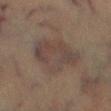Captured during whole-body skin photography for melanoma surveillance; the lesion was not biopsied.
A close-up tile cropped from a whole-body skin photograph, about 15 mm across.
A male subject, in their mid- to late 40s.
An algorithmic analysis of the crop reported an eccentricity of roughly 0.75 and two-axis asymmetry of about 0.2. The software also gave an average lesion color of about L≈39 a*≈12 b*≈20 (CIELAB), roughly 6 lightness units darker than nearby skin, and a normalized border contrast of about 6. And it measured a classifier nevus-likeness of about 5/100 and lesion-presence confidence of about 80/100.
The recorded lesion diameter is about 7 mm.
The tile uses cross-polarized illumination.
From the right lower leg.